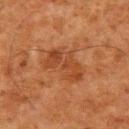Impression:
Imaged during a routine full-body skin examination; the lesion was not biopsied and no histopathology is available.
Background:
The recorded lesion diameter is about 5.5 mm. Located on the upper back. A 15 mm close-up extracted from a 3D total-body photography capture. The tile uses cross-polarized illumination. The subject is a male roughly 80 years of age.